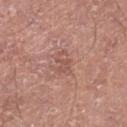The lesion-visualizer software estimated a lesion color around L≈53 a*≈22 b*≈26 in CIELAB, roughly 7 lightness units darker than nearby skin, and a lesion-to-skin contrast of about 5 (normalized; higher = more distinct). It also reported a classifier nevus-likeness of about 0/100 and a detector confidence of about 100 out of 100 that the crop contains a lesion. The recorded lesion diameter is about 2.5 mm. A male subject aged around 70. Located on the right lower leg. Cropped from a total-body skin-imaging series; the visible field is about 15 mm.